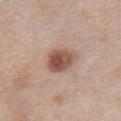Part of a total-body skin-imaging series; this lesion was reviewed on a skin check and was not flagged for biopsy.
Imaged with white-light lighting.
From the chest.
A 15 mm close-up tile from a total-body photography series done for melanoma screening.
Approximately 4 mm at its widest.
The lesion-visualizer software estimated a footprint of about 9 mm² and an outline eccentricity of about 0.7 (0 = round, 1 = elongated). The analysis additionally found a lesion color around L≈52 a*≈21 b*≈26 in CIELAB, roughly 14 lightness units darker than nearby skin, and a lesion-to-skin contrast of about 9.5 (normalized; higher = more distinct). The software also gave a within-lesion color-variation index near 5.5/10 and radial color variation of about 2. The analysis additionally found a detector confidence of about 100 out of 100 that the crop contains a lesion.
The patient is a female roughly 40 years of age.